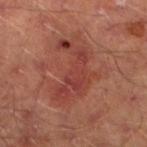Findings:
– workup · imaged on a skin check; not biopsied
– image source · ~15 mm crop, total-body skin-cancer survey
– illumination · cross-polarized
– site · the leg
– size · ~6.5 mm (longest diameter)
– subject · male, aged approximately 65